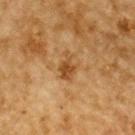The lesion was photographed on a routine skin check and not biopsied; there is no pathology result. The patient is a male in their mid-80s or older. An algorithmic analysis of the crop reported a border-irregularity rating of about 4/10, a color-variation rating of about 2.5/10, and peripheral color asymmetry of about 1. Longest diameter approximately 3 mm. The lesion is on the upper back. Imaged with cross-polarized lighting. A 15 mm close-up extracted from a 3D total-body photography capture.